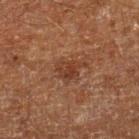This is a cross-polarized tile.
An algorithmic analysis of the crop reported an automated nevus-likeness rating near 5 out of 100.
A region of skin cropped from a whole-body photographic capture, roughly 15 mm wide.
On the right lower leg.
About 3.5 mm across.
A male subject roughly 60 years of age.